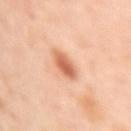Impression: The lesion was photographed on a routine skin check and not biopsied; there is no pathology result. Image and clinical context: Automated image analysis of the tile measured a lesion area of about 6 mm², a shape eccentricity near 0.7, and a symmetry-axis asymmetry near 0.2. And it measured about 13 CIELAB-L* units darker than the surrounding skin and a normalized border contrast of about 8. The analysis additionally found a border-irregularity index near 2/10, a color-variation rating of about 4/10, and radial color variation of about 1. It also reported a classifier nevus-likeness of about 95/100 and lesion-presence confidence of about 100/100. The lesion is on the left arm. A close-up tile cropped from a whole-body skin photograph, about 15 mm across. A female patient aged approximately 40. Longest diameter approximately 3.5 mm. The tile uses cross-polarized illumination.